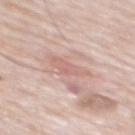Q: Is there a histopathology result?
A: total-body-photography surveillance lesion; no biopsy
Q: How was this image acquired?
A: ~15 mm tile from a whole-body skin photo
Q: Who is the patient?
A: male, aged 78 to 82
Q: How was the tile lit?
A: white-light
Q: How large is the lesion?
A: ≈4 mm
Q: Where on the body is the lesion?
A: the back